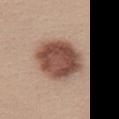Impression: Imaged during a routine full-body skin examination; the lesion was not biopsied and no histopathology is available. Background: The tile uses white-light illumination. A 15 mm close-up extracted from a 3D total-body photography capture. Located on the chest. The recorded lesion diameter is about 6 mm. The subject is a female in their mid-20s.